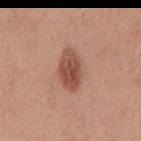Recorded during total-body skin imaging; not selected for excision or biopsy. The patient is a male aged approximately 50. A 15 mm close-up extracted from a 3D total-body photography capture. On the mid back.